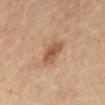The lesion was tiled from a total-body skin photograph and was not biopsied.